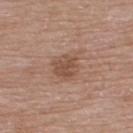Q: Was a biopsy performed?
A: total-body-photography surveillance lesion; no biopsy
Q: Lesion size?
A: ≈3.5 mm
Q: What did automated image analysis measure?
A: a lesion area of about 7 mm², an eccentricity of roughly 0.35, and two-axis asymmetry of about 0.25; a color-variation rating of about 2.5/10 and a peripheral color-asymmetry measure near 1; an automated nevus-likeness rating near 0 out of 100 and a detector confidence of about 100 out of 100 that the crop contains a lesion
Q: Where on the body is the lesion?
A: the upper back
Q: What are the patient's age and sex?
A: male, aged 73–77
Q: How was this image acquired?
A: total-body-photography crop, ~15 mm field of view